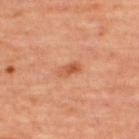Clinical impression: Recorded during total-body skin imaging; not selected for excision or biopsy. Context: The lesion is located on the upper back. The recorded lesion diameter is about 2.5 mm. A female patient, aged 43–47. Cropped from a whole-body photographic skin survey; the tile spans about 15 mm.